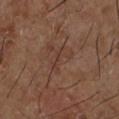This lesion was catalogued during total-body skin photography and was not selected for biopsy. The tile uses cross-polarized illumination. A male subject aged around 65. From the left lower leg. A close-up tile cropped from a whole-body skin photograph, about 15 mm across.